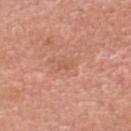Notes:
- biopsy status: total-body-photography surveillance lesion; no biopsy
- diameter: ≈2 mm
- lighting: white-light illumination
- image source: ~15 mm crop, total-body skin-cancer survey
- location: the head or neck
- image-analysis metrics: an area of roughly 1.5 mm², an outline eccentricity of about 0.8 (0 = round, 1 = elongated), and a shape-asymmetry score of about 0.4 (0 = symmetric); border irregularity of about 4 on a 0–10 scale, a within-lesion color-variation index near 0/10, and peripheral color asymmetry of about 0; a nevus-likeness score of about 0/100 and lesion-presence confidence of about 100/100
- patient: male, aged 58 to 62